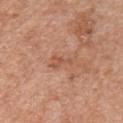Findings:
– notes — no biopsy performed (imaged during a skin exam)
– patient — male, approximately 60 years of age
– illumination — white-light
– body site — the chest
– acquisition — 15 mm crop, total-body photography
– TBP lesion metrics — a footprint of about 4.5 mm², a shape eccentricity near 0.9, and two-axis asymmetry of about 0.55; a lesion color around L≈55 a*≈24 b*≈32 in CIELAB, about 7 CIELAB-L* units darker than the surrounding skin, and a normalized border contrast of about 5; a border-irregularity index near 6/10, internal color variation of about 1.5 on a 0–10 scale, and peripheral color asymmetry of about 0.5